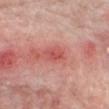This lesion was catalogued during total-body skin photography and was not selected for biopsy. A 15 mm crop from a total-body photograph taken for skin-cancer surveillance. The lesion is on the right forearm. Captured under white-light illumination. A male subject, in their mid-70s.